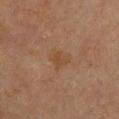Automated image analysis of the tile measured a lesion area of about 5 mm², an eccentricity of roughly 0.45, and a shape-asymmetry score of about 0.3 (0 = symmetric). The software also gave a lesion color around L≈36 a*≈16 b*≈26 in CIELAB, roughly 4 lightness units darker than nearby skin, and a normalized lesion–skin contrast near 5. The analysis additionally found a border-irregularity rating of about 3/10, a within-lesion color-variation index near 1.5/10, and radial color variation of about 0.5. The analysis additionally found a lesion-detection confidence of about 100/100.
The lesion is located on the chest.
A female subject approximately 60 years of age.
Longest diameter approximately 2.5 mm.
A 15 mm close-up extracted from a 3D total-body photography capture.